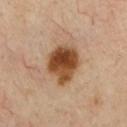No biopsy was performed on this lesion — it was imaged during a full skin examination and was not determined to be concerning. A male patient about 55 years old. A region of skin cropped from a whole-body photographic capture, roughly 15 mm wide. From the chest.